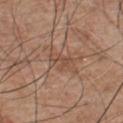Notes:
- biopsy status · no biopsy performed (imaged during a skin exam)
- size · ~3 mm (longest diameter)
- patient · male, aged approximately 55
- image · 15 mm crop, total-body photography
- automated lesion analysis · an area of roughly 3.5 mm², an eccentricity of roughly 0.8, and a shape-asymmetry score of about 0.55 (0 = symmetric); roughly 7 lightness units darker than nearby skin and a normalized border contrast of about 5.5; a within-lesion color-variation index near 0/10 and radial color variation of about 0; a classifier nevus-likeness of about 0/100 and lesion-presence confidence of about 65/100
- body site · the chest
- illumination · white-light illumination